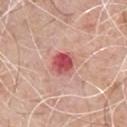The subject is a male roughly 70 years of age. From the chest. A lesion tile, about 15 mm wide, cut from a 3D total-body photograph.